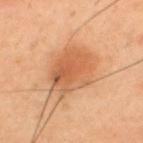Clinical impression:
Recorded during total-body skin imaging; not selected for excision or biopsy.
Image and clinical context:
Measured at roughly 6 mm in maximum diameter. This is a cross-polarized tile. A male patient, aged around 40. Cropped from a whole-body photographic skin survey; the tile spans about 15 mm. The lesion is located on the upper back. Automated image analysis of the tile measured border irregularity of about 2.5 on a 0–10 scale and peripheral color asymmetry of about 2. It also reported a detector confidence of about 100 out of 100 that the crop contains a lesion.